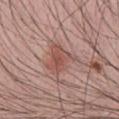biopsy status = total-body-photography surveillance lesion; no biopsy
illumination = white-light illumination
anatomic site = the front of the torso
patient = male, aged around 30
lesion size = about 4 mm
image source = 15 mm crop, total-body photography